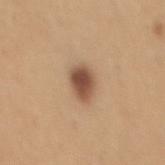This lesion was catalogued during total-body skin photography and was not selected for biopsy.
From the mid back.
An algorithmic analysis of the crop reported an area of roughly 8 mm², an outline eccentricity of about 0.7 (0 = round, 1 = elongated), and a symmetry-axis asymmetry near 0.15. It also reported a border-irregularity rating of about 1.5/10, internal color variation of about 5 on a 0–10 scale, and radial color variation of about 1.5.
The subject is a male aged around 50.
Approximately 3.5 mm at its widest.
A 15 mm crop from a total-body photograph taken for skin-cancer surveillance.